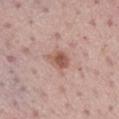Clinical impression:
Captured during whole-body skin photography for melanoma surveillance; the lesion was not biopsied.
Background:
Longest diameter approximately 3 mm. Automated tile analysis of the lesion measured an average lesion color of about L≈55 a*≈21 b*≈26 (CIELAB), roughly 11 lightness units darker than nearby skin, and a normalized lesion–skin contrast near 8. It also reported border irregularity of about 2 on a 0–10 scale, internal color variation of about 3.5 on a 0–10 scale, and peripheral color asymmetry of about 1. On the mid back. A male patient, aged 68 to 72. A 15 mm close-up tile from a total-body photography series done for melanoma screening.